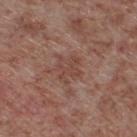Recorded during total-body skin imaging; not selected for excision or biopsy.
Located on the left lower leg.
Captured under white-light illumination.
The patient is a male aged around 55.
A 15 mm close-up extracted from a 3D total-body photography capture.
About 3 mm across.